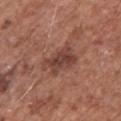{
  "biopsy_status": "not biopsied; imaged during a skin examination",
  "patient": {
    "sex": "male",
    "age_approx": 75
  },
  "image": {
    "source": "total-body photography crop",
    "field_of_view_mm": 15
  },
  "lesion_size": {
    "long_diameter_mm_approx": 4.5
  },
  "lighting": "white-light",
  "automated_metrics": {
    "area_mm2_approx": 10.0,
    "eccentricity": 0.8,
    "shape_asymmetry": 0.25,
    "border_irregularity_0_10": 3.5,
    "peripheral_color_asymmetry": 1.5
  },
  "site": "front of the torso"
}